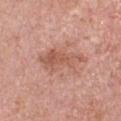Part of a total-body skin-imaging series; this lesion was reviewed on a skin check and was not flagged for biopsy.
The lesion-visualizer software estimated a footprint of about 14 mm² and a shape eccentricity near 0.9. And it measured an automated nevus-likeness rating near 5 out of 100 and a lesion-detection confidence of about 100/100.
The lesion is located on the front of the torso.
Longest diameter approximately 6.5 mm.
Cropped from a total-body skin-imaging series; the visible field is about 15 mm.
Imaged with white-light lighting.
A female subject about 40 years old.